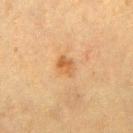Captured during whole-body skin photography for melanoma surveillance; the lesion was not biopsied. The lesion is on the chest. Imaged with cross-polarized lighting. This image is a 15 mm lesion crop taken from a total-body photograph. The lesion-visualizer software estimated a lesion color around L≈50 a*≈19 b*≈37 in CIELAB and roughly 8 lightness units darker than nearby skin. And it measured a border-irregularity index near 2/10, internal color variation of about 4 on a 0–10 scale, and peripheral color asymmetry of about 1.5. And it measured lesion-presence confidence of about 100/100. Approximately 2.5 mm at its widest. The subject is a female approximately 55 years of age.